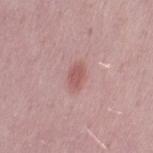The lesion was photographed on a routine skin check and not biopsied; there is no pathology result. Captured under white-light illumination. The total-body-photography lesion software estimated a shape-asymmetry score of about 0.2 (0 = symmetric). It also reported an average lesion color of about L≈56 a*≈25 b*≈22 (CIELAB) and a lesion-to-skin contrast of about 6.5 (normalized; higher = more distinct). And it measured border irregularity of about 2 on a 0–10 scale, a color-variation rating of about 1.5/10, and radial color variation of about 0.5. A female subject aged 13–17. A region of skin cropped from a whole-body photographic capture, roughly 15 mm wide. The lesion is on the left thigh. The recorded lesion diameter is about 3 mm.